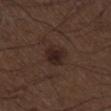Assessment: Captured during whole-body skin photography for melanoma surveillance; the lesion was not biopsied. Image and clinical context: From the left lower leg. Captured under white-light illumination. Automated image analysis of the tile measured a lesion area of about 7 mm², an eccentricity of roughly 0.55, and two-axis asymmetry of about 0.2. It also reported a nevus-likeness score of about 90/100 and lesion-presence confidence of about 100/100. The patient is a male in their 50s. A lesion tile, about 15 mm wide, cut from a 3D total-body photograph.